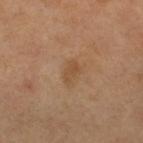Captured during whole-body skin photography for melanoma surveillance; the lesion was not biopsied. The lesion's longest dimension is about 2.5 mm. On the leg. An algorithmic analysis of the crop reported an eccentricity of roughly 0.85 and a symmetry-axis asymmetry near 0.3. And it measured a within-lesion color-variation index near 1/10 and radial color variation of about 0.5. The software also gave an automated nevus-likeness rating near 5 out of 100 and lesion-presence confidence of about 100/100. Captured under cross-polarized illumination. A female patient in their mid- to late 50s. A 15 mm close-up extracted from a 3D total-body photography capture.